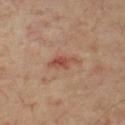Impression:
Imaged during a routine full-body skin examination; the lesion was not biopsied and no histopathology is available.
Acquisition and patient details:
The lesion's longest dimension is about 4 mm. This is a cross-polarized tile. A male subject, in their 70s. A lesion tile, about 15 mm wide, cut from a 3D total-body photograph. From the front of the torso.